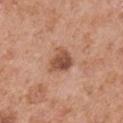<case>
<biopsy_status>not biopsied; imaged during a skin examination</biopsy_status>
<lighting>white-light</lighting>
<automated_metrics>
  <cielab_L>51</cielab_L>
  <cielab_a>23</cielab_a>
  <cielab_b>30</cielab_b>
  <vs_skin_darker_L>13.0</vs_skin_darker_L>
  <vs_skin_contrast_norm>8.5</vs_skin_contrast_norm>
  <border_irregularity_0_10>3.0</border_irregularity_0_10>
  <color_variation_0_10>4.5</color_variation_0_10>
  <peripheral_color_asymmetry>1.5</peripheral_color_asymmetry>
</automated_metrics>
<lesion_size>
  <long_diameter_mm_approx>3.5</long_diameter_mm_approx>
</lesion_size>
<patient>
  <sex>male</sex>
  <age_approx>55</age_approx>
</patient>
<site>right upper arm</site>
<image>
  <source>total-body photography crop</source>
  <field_of_view_mm>15</field_of_view_mm>
</image>
</case>